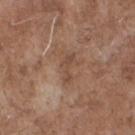Imaged during a routine full-body skin examination; the lesion was not biopsied and no histopathology is available.
A 15 mm close-up extracted from a 3D total-body photography capture.
The subject is a male about 70 years old.
The lesion is on the front of the torso.